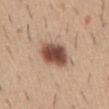workup: imaged on a skin check; not biopsied | lesion size: about 4 mm | location: the abdomen | TBP lesion metrics: a lesion area of about 12 mm², a shape eccentricity near 0.55, and two-axis asymmetry of about 0.2; border irregularity of about 2 on a 0–10 scale and a within-lesion color-variation index near 6.5/10 | imaging modality: ~15 mm crop, total-body skin-cancer survey | subject: male, in their 40s | lighting: white-light illumination.